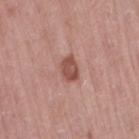Q: Was this lesion biopsied?
A: imaged on a skin check; not biopsied
Q: What are the patient's age and sex?
A: female, in their mid- to late 60s
Q: Where on the body is the lesion?
A: the right thigh
Q: Lesion size?
A: ≈3 mm
Q: What is the imaging modality?
A: ~15 mm crop, total-body skin-cancer survey
Q: Illumination type?
A: white-light